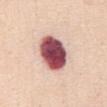Impression: The lesion was photographed on a routine skin check and not biopsied; there is no pathology result. Background: The patient is a female about 55 years old. About 5 mm across. Imaged with white-light lighting. A 15 mm close-up extracted from a 3D total-body photography capture. The lesion is located on the abdomen.